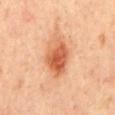Part of a total-body skin-imaging series; this lesion was reviewed on a skin check and was not flagged for biopsy.
The subject is a female in their mid-40s.
From the back.
This is a cross-polarized tile.
A 15 mm close-up tile from a total-body photography series done for melanoma screening.
The lesion's longest dimension is about 5 mm.